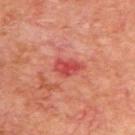{
  "biopsy_status": "not biopsied; imaged during a skin examination",
  "automated_metrics": {
    "area_mm2_approx": 5.5,
    "eccentricity": 0.8,
    "shape_asymmetry": 0.3,
    "lesion_detection_confidence_0_100": 100
  },
  "image": {
    "source": "total-body photography crop",
    "field_of_view_mm": 15
  },
  "site": "upper back",
  "lesion_size": {
    "long_diameter_mm_approx": 3.5
  },
  "lighting": "cross-polarized",
  "patient": {
    "sex": "male",
    "age_approx": 70
  }
}